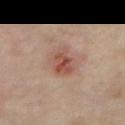Assessment: This lesion was catalogued during total-body skin photography and was not selected for biopsy. Image and clinical context: Longest diameter approximately 4.5 mm. The tile uses cross-polarized illumination. The lesion is on the right forearm. Automated image analysis of the tile measured a mean CIELAB color near L≈49 a*≈21 b*≈26, roughly 10 lightness units darker than nearby skin, and a lesion-to-skin contrast of about 7.5 (normalized; higher = more distinct). It also reported a color-variation rating of about 6.5/10. And it measured an automated nevus-likeness rating near 5 out of 100 and a lesion-detection confidence of about 100/100. A lesion tile, about 15 mm wide, cut from a 3D total-body photograph. A female subject, approximately 35 years of age.